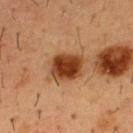Part of a total-body skin-imaging series; this lesion was reviewed on a skin check and was not flagged for biopsy.
Captured under cross-polarized illumination.
Automated image analysis of the tile measured a footprint of about 10 mm², an outline eccentricity of about 0.55 (0 = round, 1 = elongated), and a symmetry-axis asymmetry near 0.2. And it measured an automated nevus-likeness rating near 100 out of 100.
A male patient aged approximately 55.
Cropped from a total-body skin-imaging series; the visible field is about 15 mm.
On the chest.
The recorded lesion diameter is about 4 mm.